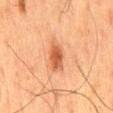Q: Was this lesion biopsied?
A: total-body-photography surveillance lesion; no biopsy
Q: Who is the patient?
A: male, in their 60s
Q: What lighting was used for the tile?
A: cross-polarized
Q: Lesion location?
A: the mid back
Q: Lesion size?
A: ≈3.5 mm
Q: What is the imaging modality?
A: ~15 mm tile from a whole-body skin photo